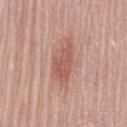{"biopsy_status": "not biopsied; imaged during a skin examination", "patient": {"sex": "female", "age_approx": 65}, "automated_metrics": {"area_mm2_approx": 11.0, "shape_asymmetry": 0.35, "cielab_L": 57, "cielab_a": 24, "cielab_b": 27, "vs_skin_contrast_norm": 6.5, "nevus_likeness_0_100": 60}, "image": {"source": "total-body photography crop", "field_of_view_mm": 15}, "lesion_size": {"long_diameter_mm_approx": 6.0}, "lighting": "white-light", "site": "lower back"}